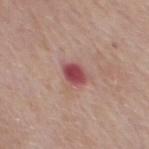<case>
  <biopsy_status>not biopsied; imaged during a skin examination</biopsy_status>
  <site>chest</site>
  <lesion_size>
    <long_diameter_mm_approx>3.0</long_diameter_mm_approx>
  </lesion_size>
  <image>
    <source>total-body photography crop</source>
    <field_of_view_mm>15</field_of_view_mm>
  </image>
  <patient>
    <sex>male</sex>
    <age_approx>70</age_approx>
  </patient>
  <automated_metrics>
    <cielab_L>47</cielab_L>
    <cielab_a>30</cielab_a>
    <cielab_b>20</cielab_b>
    <vs_skin_darker_L>14.0</vs_skin_darker_L>
    <vs_skin_contrast_norm>10.5</vs_skin_contrast_norm>
    <nevus_likeness_0_100>0</nevus_likeness_0_100>
    <lesion_detection_confidence_0_100>100</lesion_detection_confidence_0_100>
  </automated_metrics>
  <lighting>white-light</lighting>
</case>